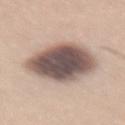Part of a total-body skin-imaging series; this lesion was reviewed on a skin check and was not flagged for biopsy. A male patient, in their 60s. A 15 mm crop from a total-body photograph taken for skin-cancer surveillance. The lesion is on the lower back.